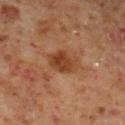No biopsy was performed on this lesion — it was imaged during a full skin examination and was not determined to be concerning. A 15 mm close-up tile from a total-body photography series done for melanoma screening. On the right lower leg. This is a cross-polarized tile. The patient is a male aged 58–62.